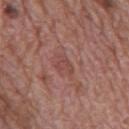The lesion was photographed on a routine skin check and not biopsied; there is no pathology result.
The subject is a male aged around 70.
The lesion is on the mid back.
Cropped from a whole-body photographic skin survey; the tile spans about 15 mm.